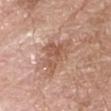Captured during whole-body skin photography for melanoma surveillance; the lesion was not biopsied. The lesion is located on the head or neck. Measured at roughly 5 mm in maximum diameter. A male patient in their mid-60s. Cropped from a total-body skin-imaging series; the visible field is about 15 mm. This is a white-light tile.